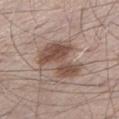| feature | finding |
|---|---|
| biopsy status | catalogued during a skin exam; not biopsied |
| acquisition | ~15 mm tile from a whole-body skin photo |
| location | the left lower leg |
| subject | male, aged around 60 |